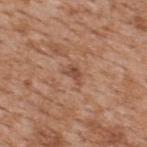Imaged during a routine full-body skin examination; the lesion was not biopsied and no histopathology is available.
Imaged with white-light lighting.
A lesion tile, about 15 mm wide, cut from a 3D total-body photograph.
The total-body-photography lesion software estimated an average lesion color of about L≈49 a*≈22 b*≈30 (CIELAB), a lesion–skin lightness drop of about 9, and a lesion-to-skin contrast of about 6.5 (normalized; higher = more distinct). The analysis additionally found a border-irregularity rating of about 4/10, internal color variation of about 1.5 on a 0–10 scale, and radial color variation of about 0.5. The analysis additionally found a lesion-detection confidence of about 100/100.
A male patient aged approximately 65.
On the upper back.